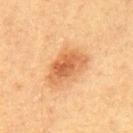Q: Is there a histopathology result?
A: no biopsy performed (imaged during a skin exam)
Q: How was this image acquired?
A: 15 mm crop, total-body photography
Q: Who is the patient?
A: male, aged 58 to 62
Q: Lesion size?
A: ~5.5 mm (longest diameter)
Q: What is the anatomic site?
A: the mid back
Q: Illumination type?
A: cross-polarized illumination